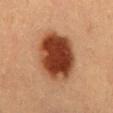Case summary:
– notes — no biopsy performed (imaged during a skin exam)
– lesion size — ≈7 mm
– imaging modality — ~15 mm crop, total-body skin-cancer survey
– automated lesion analysis — a mean CIELAB color near L≈36 a*≈24 b*≈30, a lesion–skin lightness drop of about 18, and a normalized border contrast of about 14; a classifier nevus-likeness of about 100/100 and a lesion-detection confidence of about 100/100
– illumination — cross-polarized illumination
– anatomic site — the mid back
– subject — male, about 55 years old